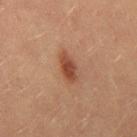Impression: The lesion was photographed on a routine skin check and not biopsied; there is no pathology result. Image and clinical context: A female subject, aged 18 to 22. Approximately 4 mm at its widest. A lesion tile, about 15 mm wide, cut from a 3D total-body photograph. Imaged with cross-polarized lighting. The lesion is on the right thigh.